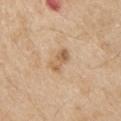Recorded during total-body skin imaging; not selected for excision or biopsy.
An algorithmic analysis of the crop reported a footprint of about 4.5 mm² and a shape-asymmetry score of about 0.2 (0 = symmetric). And it measured a border-irregularity rating of about 2.5/10 and a within-lesion color-variation index near 5/10.
The lesion is on the left upper arm.
A 15 mm close-up tile from a total-body photography series done for melanoma screening.
A male subject, about 70 years old.
Imaged with white-light lighting.